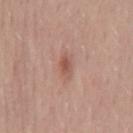Assessment:
Recorded during total-body skin imaging; not selected for excision or biopsy.
Clinical summary:
A male subject aged 53 to 57. Measured at roughly 3 mm in maximum diameter. A close-up tile cropped from a whole-body skin photograph, about 15 mm across. An algorithmic analysis of the crop reported a footprint of about 3.5 mm², an eccentricity of roughly 0.8, and a shape-asymmetry score of about 0.25 (0 = symmetric). And it measured roughly 9 lightness units darker than nearby skin. It also reported a classifier nevus-likeness of about 35/100 and lesion-presence confidence of about 100/100. Captured under white-light illumination. The lesion is on the mid back.